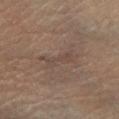Impression:
No biopsy was performed on this lesion — it was imaged during a full skin examination and was not determined to be concerning.
Context:
On the left lower leg. The tile uses cross-polarized illumination. A male patient in their mid-40s. This image is a 15 mm lesion crop taken from a total-body photograph.